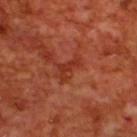* notes · total-body-photography surveillance lesion; no biopsy
* subject · male, about 70 years old
* body site · the back
* image-analysis metrics · a lesion color around L≈35 a*≈31 b*≈33 in CIELAB and a normalized lesion–skin contrast near 6; border irregularity of about 6 on a 0–10 scale, a within-lesion color-variation index near 1.5/10, and radial color variation of about 0.5; an automated nevus-likeness rating near 0 out of 100
* imaging modality · ~15 mm tile from a whole-body skin photo
* lesion diameter · ≈3.5 mm
* illumination · cross-polarized illumination